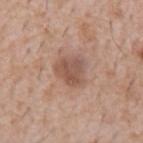<tbp_lesion>
  <image>
    <source>total-body photography crop</source>
    <field_of_view_mm>15</field_of_view_mm>
  </image>
  <lighting>white-light</lighting>
  <patient>
    <sex>male</sex>
    <age_approx>60</age_approx>
  </patient>
  <site>chest</site>
  <lesion_size>
    <long_diameter_mm_approx>3.5</long_diameter_mm_approx>
  </lesion_size>
  <automated_metrics>
    <area_mm2_approx>7.5</area_mm2_approx>
    <eccentricity>0.25</eccentricity>
    <shape_asymmetry>0.3</shape_asymmetry>
    <border_irregularity_0_10>3.0</border_irregularity_0_10>
    <color_variation_0_10>3.0</color_variation_0_10>
    <peripheral_color_asymmetry>1.0</peripheral_color_asymmetry>
    <nevus_likeness_0_100>25</nevus_likeness_0_100>
    <lesion_detection_confidence_0_100>100</lesion_detection_confidence_0_100>
  </automated_metrics>
</tbp_lesion>